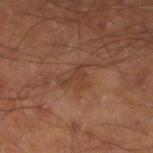| field | value |
|---|---|
| image source | 15 mm crop, total-body photography |
| automated metrics | roughly 4 lightness units darker than nearby skin and a lesion-to-skin contrast of about 4.5 (normalized; higher = more distinct); a border-irregularity rating of about 7.5/10, a color-variation rating of about 1/10, and radial color variation of about 0.5 |
| size | ~3 mm (longest diameter) |
| patient | male, aged 68–72 |
| site | the left lower leg |
| lighting | cross-polarized illumination |